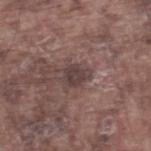Clinical summary:
The tile uses white-light illumination. On the right lower leg. The total-body-photography lesion software estimated an area of roughly 4.5 mm², an outline eccentricity of about 0.65 (0 = round, 1 = elongated), and a shape-asymmetry score of about 0.25 (0 = symmetric). And it measured a mean CIELAB color near L≈39 a*≈15 b*≈16 and a normalized lesion–skin contrast near 8.5. The subject is a male aged 73–77. A close-up tile cropped from a whole-body skin photograph, about 15 mm across. Measured at roughly 2.5 mm in maximum diameter.